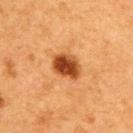Case summary:
* workup · no biopsy performed (imaged during a skin exam)
* acquisition · ~15 mm crop, total-body skin-cancer survey
* body site · the right upper arm
* diameter · about 3.5 mm
* illumination · cross-polarized illumination
* subject · female, aged 38 to 42
* automated metrics · an area of roughly 7.5 mm², an eccentricity of roughly 0.7, and two-axis asymmetry of about 0.2